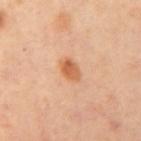Q: Was this lesion biopsied?
A: imaged on a skin check; not biopsied
Q: How was this image acquired?
A: ~15 mm crop, total-body skin-cancer survey
Q: Patient demographics?
A: male, aged 38–42
Q: What lighting was used for the tile?
A: cross-polarized
Q: What did automated image analysis measure?
A: a nevus-likeness score of about 95/100 and a lesion-detection confidence of about 100/100
Q: What is the anatomic site?
A: the right upper arm
Q: What is the lesion's diameter?
A: ~3 mm (longest diameter)